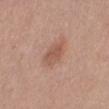Notes:
* lighting: white-light illumination
* automated lesion analysis: a footprint of about 6.5 mm²; border irregularity of about 2.5 on a 0–10 scale, a color-variation rating of about 2/10, and a peripheral color-asymmetry measure near 0.5
* site: the left lower leg
* image source: ~15 mm tile from a whole-body skin photo
* subject: female, in their mid- to late 50s